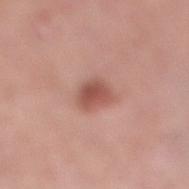Part of a total-body skin-imaging series; this lesion was reviewed on a skin check and was not flagged for biopsy.
Cropped from a total-body skin-imaging series; the visible field is about 15 mm.
Imaged with white-light lighting.
About 3.5 mm across.
On the right lower leg.
A female patient aged approximately 70.